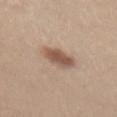{
  "biopsy_status": "not biopsied; imaged during a skin examination",
  "site": "abdomen",
  "image": {
    "source": "total-body photography crop",
    "field_of_view_mm": 15
  },
  "patient": {
    "sex": "male",
    "age_approx": 30
  }
}